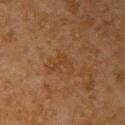{
  "biopsy_status": "not biopsied; imaged during a skin examination"
}